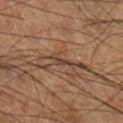Q: Is there a histopathology result?
A: catalogued during a skin exam; not biopsied
Q: Illumination type?
A: cross-polarized
Q: How was this image acquired?
A: 15 mm crop, total-body photography
Q: What is the lesion's diameter?
A: about 3.5 mm
Q: Who is the patient?
A: male, roughly 60 years of age
Q: Where on the body is the lesion?
A: the right lower leg
Q: What did automated image analysis measure?
A: a mean CIELAB color near L≈37 a*≈16 b*≈24, a lesion–skin lightness drop of about 8, and a normalized border contrast of about 8; border irregularity of about 5.5 on a 0–10 scale, internal color variation of about 0 on a 0–10 scale, and peripheral color asymmetry of about 0